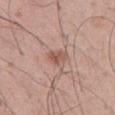Impression: Part of a total-body skin-imaging series; this lesion was reviewed on a skin check and was not flagged for biopsy. Background: Cropped from a total-body skin-imaging series; the visible field is about 15 mm. The lesion is located on the abdomen. About 2.5 mm across. Captured under white-light illumination. A male patient, aged approximately 55.